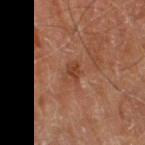The total-body-photography lesion software estimated a lesion area of about 4 mm², a shape eccentricity near 0.75, and a shape-asymmetry score of about 0.25 (0 = symmetric). It also reported internal color variation of about 3.5 on a 0–10 scale.
Cropped from a whole-body photographic skin survey; the tile spans about 15 mm.
The lesion is on the right thigh.
The lesion's longest dimension is about 2.5 mm.
Imaged with cross-polarized lighting.
A male patient, aged approximately 70.